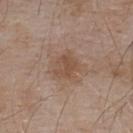Q: Was this lesion biopsied?
A: catalogued during a skin exam; not biopsied
Q: What lighting was used for the tile?
A: white-light
Q: Where on the body is the lesion?
A: the upper back
Q: How was this image acquired?
A: 15 mm crop, total-body photography
Q: What are the patient's age and sex?
A: male, aged approximately 55